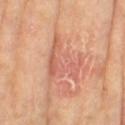The lesion was tiled from a total-body skin photograph and was not biopsied. About 6 mm across. Imaged with cross-polarized lighting. A close-up tile cropped from a whole-body skin photograph, about 15 mm across. The total-body-photography lesion software estimated an area of roughly 14 mm², an eccentricity of roughly 0.75, and a shape-asymmetry score of about 0.6 (0 = symmetric). And it measured about 8 CIELAB-L* units darker than the surrounding skin and a lesion-to-skin contrast of about 5 (normalized; higher = more distinct). It also reported a within-lesion color-variation index near 5/10 and radial color variation of about 1.5. It also reported a classifier nevus-likeness of about 0/100. The subject is a female approximately 75 years of age. The lesion is on the right thigh.